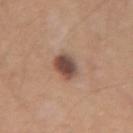Case summary:
* biopsy status — catalogued during a skin exam; not biopsied
* subject — female, in their mid- to late 40s
* image — ~15 mm tile from a whole-body skin photo
* body site — the left forearm
* image-analysis metrics — an outline eccentricity of about 0.55 (0 = round, 1 = elongated) and a symmetry-axis asymmetry near 0.2; an average lesion color of about L≈48 a*≈19 b*≈25 (CIELAB), about 15 CIELAB-L* units darker than the surrounding skin, and a lesion-to-skin contrast of about 11 (normalized; higher = more distinct); border irregularity of about 2 on a 0–10 scale, internal color variation of about 7 on a 0–10 scale, and radial color variation of about 2.5; a nevus-likeness score of about 90/100
* diameter — ≈3 mm
* lighting — white-light illumination